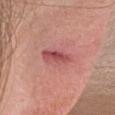Recorded during total-body skin imaging; not selected for excision or biopsy.
On the head or neck.
About 5 mm across.
An algorithmic analysis of the crop reported a footprint of about 8 mm², an outline eccentricity of about 0.9 (0 = round, 1 = elongated), and a symmetry-axis asymmetry near 0.2. And it measured a border-irregularity rating of about 2.5/10 and a peripheral color-asymmetry measure near 2.
A 15 mm close-up extracted from a 3D total-body photography capture.
A female subject, in their mid-20s.
This is a white-light tile.